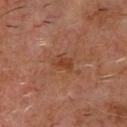<tbp_lesion>
<biopsy_status>not biopsied; imaged during a skin examination</biopsy_status>
<image>
  <source>total-body photography crop</source>
  <field_of_view_mm>15</field_of_view_mm>
</image>
<patient>
  <sex>male</sex>
  <age_approx>60</age_approx>
</patient>
<site>chest</site>
<lesion_size>
  <long_diameter_mm_approx>2.5</long_diameter_mm_approx>
</lesion_size>
<lighting>cross-polarized</lighting>
<automated_metrics>
  <area_mm2_approx>4.0</area_mm2_approx>
  <eccentricity>0.75</eccentricity>
  <shape_asymmetry>0.35</shape_asymmetry>
  <nevus_likeness_0_100>5</nevus_likeness_0_100>
  <lesion_detection_confidence_0_100>100</lesion_detection_confidence_0_100>
</automated_metrics>
</tbp_lesion>